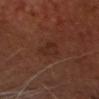Approximately 2.5 mm at its widest.
A region of skin cropped from a whole-body photographic capture, roughly 15 mm wide.
On the head or neck.
The tile uses cross-polarized illumination.
A male patient, aged 63–67.